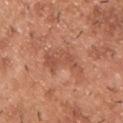This lesion was catalogued during total-body skin photography and was not selected for biopsy.
An algorithmic analysis of the crop reported a nevus-likeness score of about 0/100 and a detector confidence of about 100 out of 100 that the crop contains a lesion.
A male subject about 40 years old.
Measured at roughly 5.5 mm in maximum diameter.
A close-up tile cropped from a whole-body skin photograph, about 15 mm across.
The tile uses white-light illumination.
Located on the upper back.